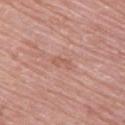Imaged during a routine full-body skin examination; the lesion was not biopsied and no histopathology is available.
A female patient, in their mid- to late 60s.
Located on the chest.
A roughly 15 mm field-of-view crop from a total-body skin photograph.